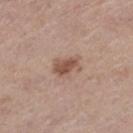The lesion was tiled from a total-body skin photograph and was not biopsied. The subject is a female about 55 years old. The lesion is on the left thigh. A region of skin cropped from a whole-body photographic capture, roughly 15 mm wide.